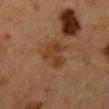The lesion was photographed on a routine skin check and not biopsied; there is no pathology result. From the chest. A female patient aged approximately 50. A close-up tile cropped from a whole-body skin photograph, about 15 mm across. About 4 mm across. Captured under cross-polarized illumination.